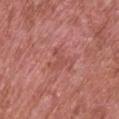Acquisition and patient details: Cropped from a whole-body photographic skin survey; the tile spans about 15 mm. Located on the upper back. The patient is a male approximately 70 years of age.